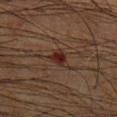The lesion was tiled from a total-body skin photograph and was not biopsied. Captured under cross-polarized illumination. A male patient, roughly 65 years of age. A roughly 15 mm field-of-view crop from a total-body skin photograph. Located on the back. About 3 mm across. Automated image analysis of the tile measured border irregularity of about 3 on a 0–10 scale, a within-lesion color-variation index near 2.5/10, and a peripheral color-asymmetry measure near 1. The software also gave a nevus-likeness score of about 0/100 and lesion-presence confidence of about 100/100.